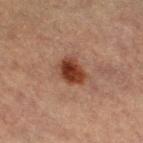Notes:
• notes: no biopsy performed (imaged during a skin exam)